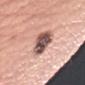| feature | finding |
|---|---|
| patient | female, aged around 60 |
| size | about 4.5 mm |
| location | the arm |
| automated metrics | a mean CIELAB color near L≈53 a*≈20 b*≈23, a lesion–skin lightness drop of about 20, and a normalized lesion–skin contrast near 13; a peripheral color-asymmetry measure near 2 |
| tile lighting | white-light |
| acquisition | ~15 mm tile from a whole-body skin photo |